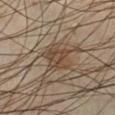Impression: Part of a total-body skin-imaging series; this lesion was reviewed on a skin check and was not flagged for biopsy. Context: A close-up tile cropped from a whole-body skin photograph, about 15 mm across. This is a cross-polarized tile. Located on the left lower leg. Automated tile analysis of the lesion measured internal color variation of about 3.5 on a 0–10 scale and peripheral color asymmetry of about 1. And it measured a classifier nevus-likeness of about 100/100 and a lesion-detection confidence of about 65/100. About 4 mm across. The subject is a male aged 38–42.